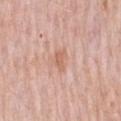Clinical impression: This lesion was catalogued during total-body skin photography and was not selected for biopsy. Acquisition and patient details: The lesion's longest dimension is about 2.5 mm. A female subject, in their mid-60s. This is a white-light tile. The lesion is located on the left forearm. A 15 mm close-up tile from a total-body photography series done for melanoma screening.